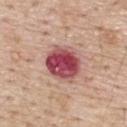• follow-up · catalogued during a skin exam; not biopsied
• acquisition · 15 mm crop, total-body photography
• tile lighting · white-light
• subject · male, in their mid- to late 50s
• TBP lesion metrics · a lesion area of about 14 mm², an eccentricity of roughly 0.15, and a symmetry-axis asymmetry near 0.1; roughly 18 lightness units darker than nearby skin and a normalized border contrast of about 13; a border-irregularity rating of about 1.5/10 and internal color variation of about 8 on a 0–10 scale
• body site · the upper back
• lesion size · ~4.5 mm (longest diameter)